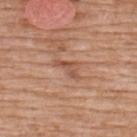notes: imaged on a skin check; not biopsied
lesion diameter: ≈3.5 mm
TBP lesion metrics: a footprint of about 4 mm², an eccentricity of roughly 0.9, and a symmetry-axis asymmetry near 0.5
anatomic site: the upper back
image: ~15 mm tile from a whole-body skin photo
patient: male, in their 60s
lighting: white-light illumination